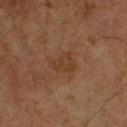Findings:
* notes: total-body-photography surveillance lesion; no biopsy
* automated lesion analysis: a footprint of about 5.5 mm² and a symmetry-axis asymmetry near 0.3; a border-irregularity rating of about 3/10, a within-lesion color-variation index near 1.5/10, and a peripheral color-asymmetry measure near 0.5
* illumination: cross-polarized
* image source: total-body-photography crop, ~15 mm field of view
* subject: male, aged 78–82
* lesion diameter: ~3.5 mm (longest diameter)
* site: the right upper arm